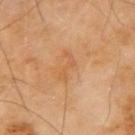Recorded during total-body skin imaging; not selected for excision or biopsy. Automated image analysis of the tile measured a footprint of about 4 mm² and an outline eccentricity of about 0.9 (0 = round, 1 = elongated). It also reported a nevus-likeness score of about 0/100 and a detector confidence of about 100 out of 100 that the crop contains a lesion. A 15 mm close-up tile from a total-body photography series done for melanoma screening. A male patient, aged 63 to 67. Located on the right upper arm.